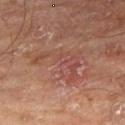Image and clinical context: The subject is a male approximately 65 years of age. Cropped from a whole-body photographic skin survey; the tile spans about 15 mm. The lesion-visualizer software estimated an area of roughly 16 mm², a shape eccentricity near 0.85, and a symmetry-axis asymmetry near 0.7. The software also gave an average lesion color of about L≈45 a*≈22 b*≈25 (CIELAB), roughly 6 lightness units darker than nearby skin, and a normalized lesion–skin contrast near 5. And it measured a color-variation rating of about 4/10 and radial color variation of about 1. And it measured a nevus-likeness score of about 0/100 and lesion-presence confidence of about 95/100. About 7 mm across. On the right thigh. This is a cross-polarized tile.